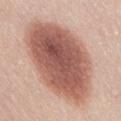follow-up: no biopsy performed (imaged during a skin exam) | patient: female, in their mid- to late 40s | lighting: white-light | site: the lower back | image-analysis metrics: a lesion color around L≈56 a*≈23 b*≈27 in CIELAB, about 17 CIELAB-L* units darker than the surrounding skin, and a normalized lesion–skin contrast near 10.5; a border-irregularity index near 1.5/10 and peripheral color asymmetry of about 2; a nevus-likeness score of about 100/100 | image: total-body-photography crop, ~15 mm field of view.